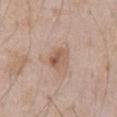Part of a total-body skin-imaging series; this lesion was reviewed on a skin check and was not flagged for biopsy.
The subject is a male approximately 60 years of age.
The tile uses white-light illumination.
Approximately 3 mm at its widest.
The lesion-visualizer software estimated a lesion color around L≈56 a*≈19 b*≈29 in CIELAB, about 9 CIELAB-L* units darker than the surrounding skin, and a lesion-to-skin contrast of about 7 (normalized; higher = more distinct).
A lesion tile, about 15 mm wide, cut from a 3D total-body photograph.
Located on the abdomen.